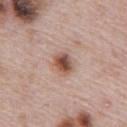Impression:
The lesion was photographed on a routine skin check and not biopsied; there is no pathology result.
Context:
A 15 mm close-up extracted from a 3D total-body photography capture. From the abdomen. A male subject aged around 70. Captured under white-light illumination. Measured at roughly 2.5 mm in maximum diameter.